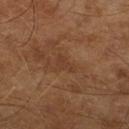Clinical impression:
Part of a total-body skin-imaging series; this lesion was reviewed on a skin check and was not flagged for biopsy.
Background:
The patient is a male aged 63–67. A 15 mm close-up extracted from a 3D total-body photography capture.